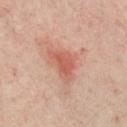Assessment:
The lesion was tiled from a total-body skin photograph and was not biopsied.
Acquisition and patient details:
The lesion-visualizer software estimated a lesion area of about 7 mm², an outline eccentricity of about 0.75 (0 = round, 1 = elongated), and a symmetry-axis asymmetry near 0.45. The software also gave a lesion color around L≈57 a*≈27 b*≈30 in CIELAB, a lesion–skin lightness drop of about 9, and a lesion-to-skin contrast of about 6.5 (normalized; higher = more distinct). And it measured a border-irregularity rating of about 4.5/10, a within-lesion color-variation index near 3/10, and radial color variation of about 1. It also reported a detector confidence of about 100 out of 100 that the crop contains a lesion. The subject is aged around 55. Imaged with cross-polarized lighting. The lesion is on the chest. A 15 mm close-up extracted from a 3D total-body photography capture.